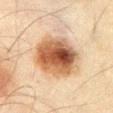Background:
The lesion is located on the abdomen. A male subject, aged around 45. A 15 mm crop from a total-body photograph taken for skin-cancer surveillance.